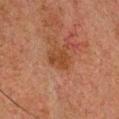The lesion was tiled from a total-body skin photograph and was not biopsied.
The patient is a male about 60 years old.
An algorithmic analysis of the crop reported an area of roughly 3.5 mm² and an eccentricity of roughly 0.75.
Measured at roughly 2.5 mm in maximum diameter.
A 15 mm crop from a total-body photograph taken for skin-cancer surveillance.
On the head or neck.
Imaged with cross-polarized lighting.